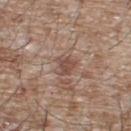From the upper back. A male subject about 65 years old. A lesion tile, about 15 mm wide, cut from a 3D total-body photograph. Longest diameter approximately 3 mm.